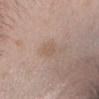follow-up: catalogued during a skin exam; not biopsied | lesion diameter: about 2.5 mm | imaging modality: ~15 mm tile from a whole-body skin photo | body site: the head or neck | subject: female, aged 23–27 | image-analysis metrics: a footprint of about 3 mm², a shape eccentricity near 0.75, and a shape-asymmetry score of about 0.3 (0 = symmetric); an average lesion color of about L≈57 a*≈16 b*≈27 (CIELAB); a border-irregularity rating of about 2.5/10; a nevus-likeness score of about 0/100 and a lesion-detection confidence of about 100/100.